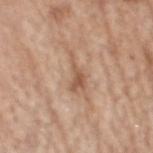No biopsy was performed on this lesion — it was imaged during a full skin examination and was not determined to be concerning. A roughly 15 mm field-of-view crop from a total-body skin photograph. On the head or neck. The tile uses white-light illumination. A male patient, aged 68–72. Automated tile analysis of the lesion measured a lesion area of about 5 mm² and a symmetry-axis asymmetry near 0.6.